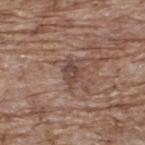biopsy status: imaged on a skin check; not biopsied | subject: male, aged approximately 70 | diameter: ~3.5 mm (longest diameter) | tile lighting: white-light | body site: the upper back | image: ~15 mm crop, total-body skin-cancer survey.